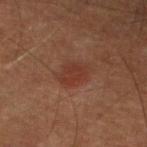Part of a total-body skin-imaging series; this lesion was reviewed on a skin check and was not flagged for biopsy. The lesion-visualizer software estimated border irregularity of about 2.5 on a 0–10 scale, a color-variation rating of about 2.5/10, and radial color variation of about 1. This is a cross-polarized tile. The lesion is located on the left lower leg. A 15 mm close-up tile from a total-body photography series done for melanoma screening. A male subject in their 60s. About 4 mm across.